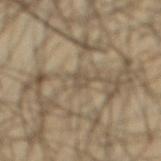Part of a total-body skin-imaging series; this lesion was reviewed on a skin check and was not flagged for biopsy.
Cropped from a total-body skin-imaging series; the visible field is about 15 mm.
The lesion is on the abdomen.
A male patient, about 40 years old.